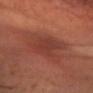Recorded during total-body skin imaging; not selected for excision or biopsy.
From the head or neck.
About 6.5 mm across.
This image is a 15 mm lesion crop taken from a total-body photograph.
Automated tile analysis of the lesion measured an area of roughly 18 mm² and a symmetry-axis asymmetry near 0.3. The analysis additionally found internal color variation of about 3.5 on a 0–10 scale and a peripheral color-asymmetry measure near 1. The software also gave a classifier nevus-likeness of about 0/100 and lesion-presence confidence of about 80/100.
The tile uses cross-polarized illumination.
A male patient, roughly 60 years of age.